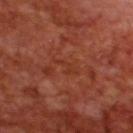Cropped from a total-body skin-imaging series; the visible field is about 15 mm.
This is a cross-polarized tile.
The lesion is located on the upper back.
The patient is a male aged approximately 70.
About 3 mm across.